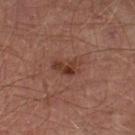biopsy_status: not biopsied; imaged during a skin examination
site: left lower leg
image:
  source: total-body photography crop
  field_of_view_mm: 15
lesion_size:
  long_diameter_mm_approx: 3.5
automated_metrics:
  cielab_L: 36
  cielab_a: 22
  cielab_b: 26
  vs_skin_contrast_norm: 7.5
  nevus_likeness_0_100: 60
  lesion_detection_confidence_0_100: 100
patient:
  sex: male
  age_approx: 65
lighting: cross-polarized